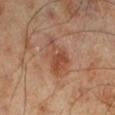workup: imaged on a skin check; not biopsied | anatomic site: the leg | tile lighting: cross-polarized illumination | acquisition: ~15 mm tile from a whole-body skin photo | patient: male, about 70 years old.